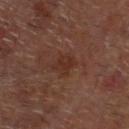notes — no biopsy performed (imaged during a skin exam)
anatomic site — the head or neck
imaging modality — ~15 mm tile from a whole-body skin photo
subject — male, roughly 60 years of age
lighting — cross-polarized illumination
lesion size — about 3 mm